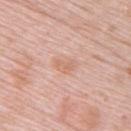follow-up=catalogued during a skin exam; not biopsied
lighting=white-light
location=the upper back
patient=female, aged 63 to 67
image=total-body-photography crop, ~15 mm field of view
automated metrics=an area of roughly 4 mm², a shape eccentricity near 0.8, and a symmetry-axis asymmetry near 0.35; a classifier nevus-likeness of about 0/100 and a detector confidence of about 100 out of 100 that the crop contains a lesion
size=≈3 mm